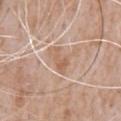Assessment:
No biopsy was performed on this lesion — it was imaged during a full skin examination and was not determined to be concerning.
Clinical summary:
A roughly 15 mm field-of-view crop from a total-body skin photograph. The total-body-photography lesion software estimated an average lesion color of about L≈60 a*≈19 b*≈31 (CIELAB), roughly 8 lightness units darker than nearby skin, and a normalized border contrast of about 6. Approximately 3.5 mm at its widest. The patient is a male aged around 65. The lesion is on the chest. Captured under white-light illumination.